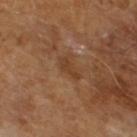Part of a total-body skin-imaging series; this lesion was reviewed on a skin check and was not flagged for biopsy. Measured at roughly 3 mm in maximum diameter. The subject is a male in their mid- to late 60s. Cropped from a total-body skin-imaging series; the visible field is about 15 mm.